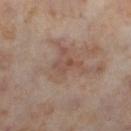Captured during whole-body skin photography for melanoma surveillance; the lesion was not biopsied.
Automated image analysis of the tile measured a border-irregularity rating of about 4.5/10, a within-lesion color-variation index near 0/10, and a peripheral color-asymmetry measure near 0. The analysis additionally found a classifier nevus-likeness of about 0/100 and a detector confidence of about 100 out of 100 that the crop contains a lesion.
Captured under cross-polarized illumination.
The recorded lesion diameter is about 3 mm.
The lesion is on the left lower leg.
A female subject in their mid- to late 50s.
A 15 mm close-up tile from a total-body photography series done for melanoma screening.